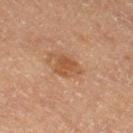Q: Is there a histopathology result?
A: no biopsy performed (imaged during a skin exam)
Q: Lesion location?
A: the right thigh
Q: Illumination type?
A: cross-polarized illumination
Q: Automated lesion metrics?
A: an average lesion color of about L≈45 a*≈19 b*≈32 (CIELAB) and a lesion–skin lightness drop of about 7; a border-irregularity rating of about 3/10, a color-variation rating of about 2.5/10, and peripheral color asymmetry of about 1; a classifier nevus-likeness of about 40/100 and a detector confidence of about 100 out of 100 that the crop contains a lesion
Q: What kind of image is this?
A: 15 mm crop, total-body photography
Q: Patient demographics?
A: female, in their mid-50s